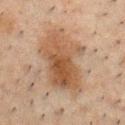{
  "biopsy_status": "not biopsied; imaged during a skin examination"
}